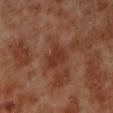Part of a total-body skin-imaging series; this lesion was reviewed on a skin check and was not flagged for biopsy.
From the left lower leg.
A roughly 15 mm field-of-view crop from a total-body skin photograph.
The subject is a male aged 68–72.